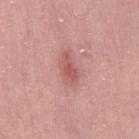{
  "biopsy_status": "not biopsied; imaged during a skin examination",
  "image": {
    "source": "total-body photography crop",
    "field_of_view_mm": 15
  },
  "patient": {
    "sex": "female",
    "age_approx": 60
  },
  "site": "right thigh"
}